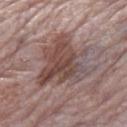The lesion was photographed on a routine skin check and not biopsied; there is no pathology result. The patient is a female approximately 85 years of age or older. A 15 mm close-up tile from a total-body photography series done for melanoma screening. The lesion is on the right thigh. Imaged with white-light lighting. The lesion's longest dimension is about 7 mm. Automated tile analysis of the lesion measured a lesion-to-skin contrast of about 8 (normalized; higher = more distinct). The software also gave border irregularity of about 5 on a 0–10 scale, a within-lesion color-variation index near 7.5/10, and radial color variation of about 2.5.